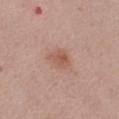biopsy status = imaged on a skin check; not biopsied | location = the left thigh | automated lesion analysis = a lesion color around L≈55 a*≈21 b*≈27 in CIELAB, a lesion–skin lightness drop of about 8, and a normalized lesion–skin contrast near 6; a classifier nevus-likeness of about 35/100 | imaging modality = total-body-photography crop, ~15 mm field of view | patient = female, aged around 40 | lesion size = ~3 mm (longest diameter).